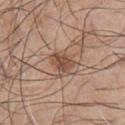Part of a total-body skin-imaging series; this lesion was reviewed on a skin check and was not flagged for biopsy.
The lesion's longest dimension is about 3 mm.
On the chest.
A 15 mm close-up tile from a total-body photography series done for melanoma screening.
Automated image analysis of the tile measured an area of roughly 5 mm², an eccentricity of roughly 0.65, and a symmetry-axis asymmetry near 0.2. It also reported an average lesion color of about L≈49 a*≈20 b*≈28 (CIELAB), roughly 11 lightness units darker than nearby skin, and a normalized border contrast of about 8. The analysis additionally found a classifier nevus-likeness of about 70/100 and a detector confidence of about 100 out of 100 that the crop contains a lesion.
A male patient, aged 43 to 47.